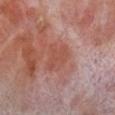notes = no biopsy performed (imaged during a skin exam) | image = ~15 mm crop, total-body skin-cancer survey | patient = male, roughly 70 years of age | size = ~3.5 mm (longest diameter) | TBP lesion metrics = a color-variation rating of about 2/10 and a peripheral color-asymmetry measure near 0.5; a classifier nevus-likeness of about 0/100 and a detector confidence of about 100 out of 100 that the crop contains a lesion | body site = the right lower leg | lighting = cross-polarized.